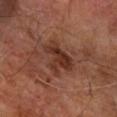follow-up — imaged on a skin check; not biopsied
illumination — cross-polarized
image — ~15 mm crop, total-body skin-cancer survey
site — the right forearm
patient — male, aged 68–72
TBP lesion metrics — an average lesion color of about L≈32 a*≈22 b*≈26 (CIELAB), a lesion–skin lightness drop of about 9, and a normalized border contrast of about 8.5; a border-irregularity index near 6/10 and a within-lesion color-variation index near 3/10
lesion size — ~4.5 mm (longest diameter)